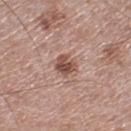Captured during whole-body skin photography for melanoma surveillance; the lesion was not biopsied. A region of skin cropped from a whole-body photographic capture, roughly 15 mm wide. From the right lower leg. A male patient about 50 years old.